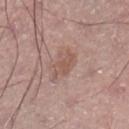  biopsy_status: not biopsied; imaged during a skin examination
  automated_metrics:
    cielab_L: 54
    cielab_a: 19
    cielab_b: 25
    vs_skin_darker_L: 7.0
    vs_skin_contrast_norm: 5.5
    border_irregularity_0_10: 3.0
    color_variation_0_10: 2.0
    peripheral_color_asymmetry: 1.0
  site: right lower leg
  lesion_size:
    long_diameter_mm_approx: 3.5
  patient:
    sex: male
    age_approx: 50
  lighting: white-light
  image:
    source: total-body photography crop
    field_of_view_mm: 15